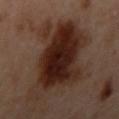Assessment:
Captured during whole-body skin photography for melanoma surveillance; the lesion was not biopsied.
Acquisition and patient details:
An algorithmic analysis of the crop reported a footprint of about 46 mm², an eccentricity of roughly 0.65, and a symmetry-axis asymmetry near 0.3. The analysis additionally found a classifier nevus-likeness of about 95/100 and a lesion-detection confidence of about 100/100. The subject is a female in their 40s. The recorded lesion diameter is about 9 mm. The lesion is on the left arm. A roughly 15 mm field-of-view crop from a total-body skin photograph.